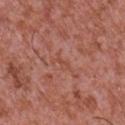Clinical impression: The lesion was tiled from a total-body skin photograph and was not biopsied. Acquisition and patient details: This image is a 15 mm lesion crop taken from a total-body photograph. This is a white-light tile. Approximately 2.5 mm at its widest. Automated tile analysis of the lesion measured an average lesion color of about L≈49 a*≈26 b*≈30 (CIELAB), a lesion–skin lightness drop of about 5, and a normalized lesion–skin contrast near 4.5. It also reported border irregularity of about 5 on a 0–10 scale, internal color variation of about 0 on a 0–10 scale, and peripheral color asymmetry of about 0. The analysis additionally found lesion-presence confidence of about 80/100. The lesion is located on the chest. A male subject, about 45 years old.